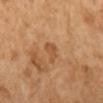  biopsy_status: not biopsied; imaged during a skin examination
  site: leg
  lesion_size:
    long_diameter_mm_approx: 2.5
  image:
    source: total-body photography crop
    field_of_view_mm: 15
  patient:
    sex: female
    age_approx: 50